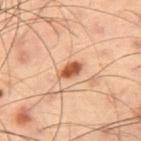Notes:
• notes: imaged on a skin check; not biopsied
• site: the leg
• patient: male, in their mid- to late 50s
• size: about 2.5 mm
• acquisition: ~15 mm tile from a whole-body skin photo